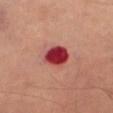Assessment: No biopsy was performed on this lesion — it was imaged during a full skin examination and was not determined to be concerning. Image and clinical context: This is a cross-polarized tile. A female subject aged 58–62. From the abdomen. The lesion's longest dimension is about 3.5 mm. Cropped from a whole-body photographic skin survey; the tile spans about 15 mm.